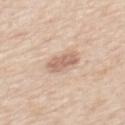{"biopsy_status": "not biopsied; imaged during a skin examination", "patient": {"sex": "male", "age_approx": 60}, "site": "back", "lighting": "white-light", "lesion_size": {"long_diameter_mm_approx": 3.5}, "image": {"source": "total-body photography crop", "field_of_view_mm": 15}}